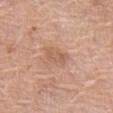Q: What is the lesion's diameter?
A: ~3 mm (longest diameter)
Q: What kind of image is this?
A: total-body-photography crop, ~15 mm field of view
Q: Lesion location?
A: the left thigh
Q: Patient demographics?
A: female, aged approximately 60